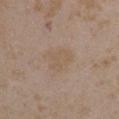follow-up = no biopsy performed (imaged during a skin exam); TBP lesion metrics = a border-irregularity rating of about 3.5/10, internal color variation of about 1.5 on a 0–10 scale, and a peripheral color-asymmetry measure near 0.5; lighting = white-light; body site = the right upper arm; patient = female, aged around 35; acquisition = total-body-photography crop, ~15 mm field of view.